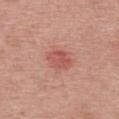This is a white-light tile. Longest diameter approximately 3 mm. The lesion is located on the back. A lesion tile, about 15 mm wide, cut from a 3D total-body photograph. A female patient, aged 63 to 67.